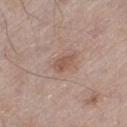size: ≈2.5 mm
tile lighting: white-light illumination
image: total-body-photography crop, ~15 mm field of view
patient: male, about 65 years old
anatomic site: the leg